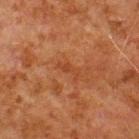{
  "biopsy_status": "not biopsied; imaged during a skin examination",
  "patient": {
    "sex": "male",
    "age_approx": 80
  },
  "site": "upper back",
  "lesion_size": {
    "long_diameter_mm_approx": 3.0
  },
  "automated_metrics": {
    "area_mm2_approx": 2.5,
    "eccentricity": 0.9,
    "shape_asymmetry": 0.35,
    "cielab_L": 34,
    "cielab_a": 23,
    "cielab_b": 31,
    "vs_skin_contrast_norm": 5.0,
    "color_variation_0_10": 0.0,
    "peripheral_color_asymmetry": 0.0,
    "nevus_likeness_0_100": 0
  },
  "image": {
    "source": "total-body photography crop",
    "field_of_view_mm": 15
  },
  "lighting": "cross-polarized"
}